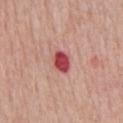follow-up: no biopsy performed (imaged during a skin exam) | image-analysis metrics: an area of roughly 5 mm² and a shape eccentricity near 0.65; roughly 17 lightness units darker than nearby skin and a normalized lesion–skin contrast near 11.5; internal color variation of about 3.5 on a 0–10 scale and a peripheral color-asymmetry measure near 1.5; a classifier nevus-likeness of about 0/100 and a detector confidence of about 100 out of 100 that the crop contains a lesion | lesion size: about 3 mm | subject: male, approximately 60 years of age | site: the chest | tile lighting: white-light | image: ~15 mm crop, total-body skin-cancer survey.